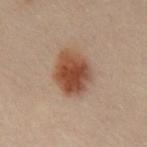The lesion was tiled from a total-body skin photograph and was not biopsied.